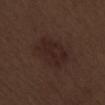The lesion was tiled from a total-body skin photograph and was not biopsied.
A male subject in their 70s.
This is a white-light tile.
Located on the arm.
A roughly 15 mm field-of-view crop from a total-body skin photograph.
The recorded lesion diameter is about 6 mm.